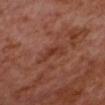Part of a total-body skin-imaging series; this lesion was reviewed on a skin check and was not flagged for biopsy. The tile uses cross-polarized illumination. An algorithmic analysis of the crop reported an area of roughly 4 mm², an eccentricity of roughly 0.8, and a symmetry-axis asymmetry near 0.4. The analysis additionally found a border-irregularity index near 4/10, a color-variation rating of about 2/10, and peripheral color asymmetry of about 0.5. Measured at roughly 3 mm in maximum diameter. A region of skin cropped from a whole-body photographic capture, roughly 15 mm wide. A male patient roughly 55 years of age. The lesion is located on the head or neck.